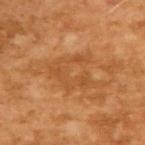notes = imaged on a skin check; not biopsied
subject = male, aged 63 to 67
lighting = cross-polarized
image = ~15 mm tile from a whole-body skin photo